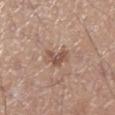The lesion was tiled from a total-body skin photograph and was not biopsied.
About 3.5 mm across.
This is a white-light tile.
Located on the right lower leg.
A male patient, aged 38 to 42.
A 15 mm crop from a total-body photograph taken for skin-cancer surveillance.